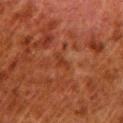Q: Was this lesion biopsied?
A: catalogued during a skin exam; not biopsied
Q: How was this image acquired?
A: ~15 mm crop, total-body skin-cancer survey
Q: What is the lesion's diameter?
A: about 2.5 mm
Q: Who is the patient?
A: male, aged around 80
Q: What is the anatomic site?
A: the leg
Q: Illumination type?
A: cross-polarized illumination